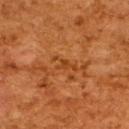  biopsy_status: not biopsied; imaged during a skin examination
  lesion_size:
    long_diameter_mm_approx: 3.5
  site: upper back
  patient:
    sex: female
    age_approx: 50
  image:
    source: total-body photography crop
    field_of_view_mm: 15
  lighting: cross-polarized